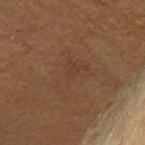This image is a 15 mm lesion crop taken from a total-body photograph. From the right upper arm. A female subject roughly 80 years of age.